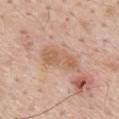follow-up = no biopsy performed (imaged during a skin exam) | lesion diameter = about 5 mm | imaging modality = ~15 mm crop, total-body skin-cancer survey | patient = male, approximately 55 years of age | lighting = white-light illumination | location = the mid back.